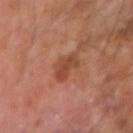Q: Is there a histopathology result?
A: catalogued during a skin exam; not biopsied
Q: Who is the patient?
A: male, aged around 60
Q: What is the imaging modality?
A: 15 mm crop, total-body photography
Q: What lighting was used for the tile?
A: cross-polarized illumination
Q: Lesion location?
A: the arm